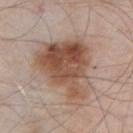Assessment: Recorded during total-body skin imaging; not selected for excision or biopsy. Context: A 15 mm close-up tile from a total-body photography series done for melanoma screening. Automated tile analysis of the lesion measured a mean CIELAB color near L≈51 a*≈19 b*≈28 and a normalized border contrast of about 10. The analysis additionally found a border-irregularity rating of about 4.5/10 and radial color variation of about 2.5. The analysis additionally found a classifier nevus-likeness of about 90/100 and lesion-presence confidence of about 100/100. The tile uses white-light illumination. The patient is a male aged approximately 55. From the chest. The recorded lesion diameter is about 8 mm.